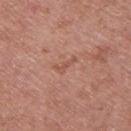<case>
  <biopsy_status>not biopsied; imaged during a skin examination</biopsy_status>
  <image>
    <source>total-body photography crop</source>
    <field_of_view_mm>15</field_of_view_mm>
  </image>
  <automated_metrics>
    <area_mm2_approx>2.5</area_mm2_approx>
    <eccentricity>0.95</eccentricity>
    <shape_asymmetry>0.55</shape_asymmetry>
    <color_variation_0_10>0.0</color_variation_0_10>
    <peripheral_color_asymmetry>0.0</peripheral_color_asymmetry>
    <nevus_likeness_0_100>0</nevus_likeness_0_100>
  </automated_metrics>
  <lighting>white-light</lighting>
  <site>back</site>
  <patient>
    <sex>female</sex>
    <age_approx>40</age_approx>
  </patient>
  <lesion_size>
    <long_diameter_mm_approx>3.0</long_diameter_mm_approx>
  </lesion_size>
</case>